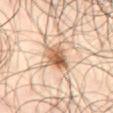biopsy_status: not biopsied; imaged during a skin examination
patient:
  sex: male
  age_approx: 65
site: abdomen
image:
  source: total-body photography crop
  field_of_view_mm: 15